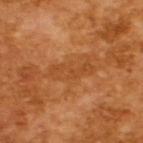No biopsy was performed on this lesion — it was imaged during a full skin examination and was not determined to be concerning. A male patient, in their mid- to late 60s. Imaged with cross-polarized lighting. Approximately 5 mm at its widest. A region of skin cropped from a whole-body photographic capture, roughly 15 mm wide.